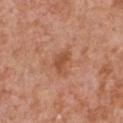<tbp_lesion>
<lighting>white-light</lighting>
<lesion_size>
  <long_diameter_mm_approx>3.0</long_diameter_mm_approx>
</lesion_size>
<site>chest</site>
<patient>
  <sex>male</sex>
  <age_approx>65</age_approx>
</patient>
<image>
  <source>total-body photography crop</source>
  <field_of_view_mm>15</field_of_view_mm>
</image>
</tbp_lesion>